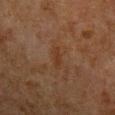The lesion was photographed on a routine skin check and not biopsied; there is no pathology result. A male patient approximately 50 years of age. The lesion is located on the chest. This is a cross-polarized tile. Cropped from a total-body skin-imaging series; the visible field is about 15 mm.